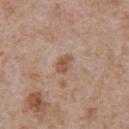Captured during whole-body skin photography for melanoma surveillance; the lesion was not biopsied. About 2.5 mm across. From the chest. The lesion-visualizer software estimated an average lesion color of about L≈53 a*≈18 b*≈29 (CIELAB), about 9 CIELAB-L* units darker than the surrounding skin, and a lesion-to-skin contrast of about 7.5 (normalized; higher = more distinct). It also reported a nevus-likeness score of about 5/100 and a detector confidence of about 100 out of 100 that the crop contains a lesion. A region of skin cropped from a whole-body photographic capture, roughly 15 mm wide. The subject is a male about 65 years old. This is a white-light tile.